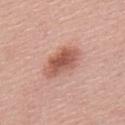No biopsy was performed on this lesion — it was imaged during a full skin examination and was not determined to be concerning.
A male patient roughly 60 years of age.
On the back.
A region of skin cropped from a whole-body photographic capture, roughly 15 mm wide.